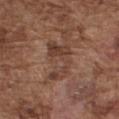Captured during whole-body skin photography for melanoma surveillance; the lesion was not biopsied.
The patient is a male in their mid- to late 70s.
The recorded lesion diameter is about 5 mm.
A 15 mm crop from a total-body photograph taken for skin-cancer surveillance.
Located on the chest.
Imaged with white-light lighting.